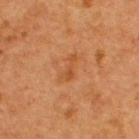Case summary:
– workup: total-body-photography surveillance lesion; no biopsy
– patient: female, aged around 40
– location: the upper back
– imaging modality: 15 mm crop, total-body photography
– lighting: cross-polarized illumination
– size: about 3 mm
– automated lesion analysis: a lesion area of about 3.5 mm², a shape eccentricity near 0.9, and two-axis asymmetry of about 0.45; an average lesion color of about L≈44 a*≈24 b*≈36 (CIELAB) and a normalized border contrast of about 5.5; an automated nevus-likeness rating near 0 out of 100 and a detector confidence of about 100 out of 100 that the crop contains a lesion